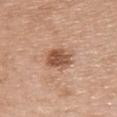biopsy_status: not biopsied; imaged during a skin examination
image:
  source: total-body photography crop
  field_of_view_mm: 15
patient:
  sex: male
  age_approx: 40
site: chest